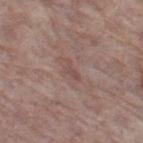Captured under white-light illumination. The lesion is located on the left thigh. A region of skin cropped from a whole-body photographic capture, roughly 15 mm wide. A female subject, in their mid- to late 80s or older.